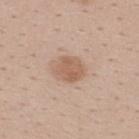No biopsy was performed on this lesion — it was imaged during a full skin examination and was not determined to be concerning. Approximately 3.5 mm at its widest. An algorithmic analysis of the crop reported a mean CIELAB color near L≈59 a*≈19 b*≈30, roughly 9 lightness units darker than nearby skin, and a normalized lesion–skin contrast near 7. The analysis additionally found a peripheral color-asymmetry measure near 1. The software also gave a nevus-likeness score of about 60/100 and a detector confidence of about 100 out of 100 that the crop contains a lesion. Imaged with white-light lighting. A male subject, aged approximately 40. A 15 mm crop from a total-body photograph taken for skin-cancer surveillance. Located on the upper back.